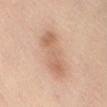illumination — white-light illumination | lesion size — about 7 mm | site — the lower back | patient — female, in their mid-50s | automated lesion analysis — a lesion area of about 13 mm², an eccentricity of roughly 0.95, and a shape-asymmetry score of about 0.15 (0 = symmetric); a mean CIELAB color near L≈64 a*≈20 b*≈32 and a lesion-to-skin contrast of about 6.5 (normalized; higher = more distinct); an automated nevus-likeness rating near 35 out of 100 and lesion-presence confidence of about 100/100 | acquisition — ~15 mm crop, total-body skin-cancer survey.